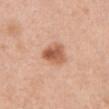No biopsy was performed on this lesion — it was imaged during a full skin examination and was not determined to be concerning.
Measured at roughly 3.5 mm in maximum diameter.
This is a white-light tile.
The total-body-photography lesion software estimated an eccentricity of roughly 0.6 and a symmetry-axis asymmetry near 0.3. And it measured a mean CIELAB color near L≈59 a*≈24 b*≈33, about 13 CIELAB-L* units darker than the surrounding skin, and a normalized border contrast of about 8.5. It also reported border irregularity of about 2.5 on a 0–10 scale and peripheral color asymmetry of about 2.5. The software also gave a classifier nevus-likeness of about 95/100 and a detector confidence of about 100 out of 100 that the crop contains a lesion.
On the front of the torso.
Cropped from a whole-body photographic skin survey; the tile spans about 15 mm.
A female subject, aged approximately 40.